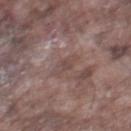workup: imaged on a skin check; not biopsied | lesion size: about 3 mm | TBP lesion metrics: a lesion area of about 4 mm², an outline eccentricity of about 0.8 (0 = round, 1 = elongated), and a symmetry-axis asymmetry near 0.25 | body site: the right thigh | illumination: white-light | patient: male, aged around 75 | image: ~15 mm crop, total-body skin-cancer survey.